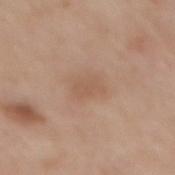Assessment:
The lesion was tiled from a total-body skin photograph and was not biopsied.
Context:
Automated tile analysis of the lesion measured a lesion–skin lightness drop of about 6 and a lesion-to-skin contrast of about 4.5 (normalized; higher = more distinct). The analysis additionally found a border-irregularity rating of about 2.5/10 and a color-variation rating of about 1.5/10. It also reported a classifier nevus-likeness of about 0/100 and a lesion-detection confidence of about 100/100. Imaged with white-light lighting. A male patient aged around 65. The lesion is located on the mid back. Longest diameter approximately 3 mm. A 15 mm crop from a total-body photograph taken for skin-cancer surveillance.